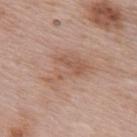notes: total-body-photography surveillance lesion; no biopsy | subject: female, roughly 65 years of age | imaging modality: 15 mm crop, total-body photography | lighting: white-light | automated lesion analysis: a footprint of about 14 mm², an outline eccentricity of about 0.75 (0 = round, 1 = elongated), and two-axis asymmetry of about 0.5; a classifier nevus-likeness of about 0/100 | diameter: ≈6 mm | anatomic site: the upper back.